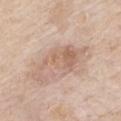The lesion was tiled from a total-body skin photograph and was not biopsied. The total-body-photography lesion software estimated a lesion area of about 15 mm², an outline eccentricity of about 0.9 (0 = round, 1 = elongated), and two-axis asymmetry of about 0.55. And it measured a border-irregularity index near 8/10, internal color variation of about 4 on a 0–10 scale, and peripheral color asymmetry of about 1. This is a white-light tile. A close-up tile cropped from a whole-body skin photograph, about 15 mm across. A male patient aged approximately 70. The lesion is located on the chest. Longest diameter approximately 7.5 mm.